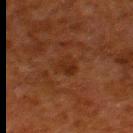site — the left lower leg
subject — male, aged 78 to 82
lesion diameter — about 3 mm
image — 15 mm crop, total-body photography
tile lighting — cross-polarized illumination